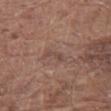This lesion was catalogued during total-body skin photography and was not selected for biopsy.
The lesion is on the left thigh.
Cropped from a total-body skin-imaging series; the visible field is about 15 mm.
A male subject aged around 80.